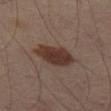Captured during whole-body skin photography for melanoma surveillance; the lesion was not biopsied. Automated tile analysis of the lesion measured a lesion area of about 13 mm², a shape eccentricity near 0.85, and two-axis asymmetry of about 0.15. The software also gave a mean CIELAB color near L≈30 a*≈15 b*≈20, a lesion–skin lightness drop of about 10, and a normalized border contrast of about 10. Captured under cross-polarized illumination. A male subject, in their 50s. Cropped from a total-body skin-imaging series; the visible field is about 15 mm. The lesion is located on the left thigh.